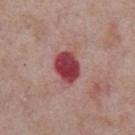Assessment: Recorded during total-body skin imaging; not selected for excision or biopsy. Image and clinical context: The lesion-visualizer software estimated border irregularity of about 1.5 on a 0–10 scale, a color-variation rating of about 4/10, and a peripheral color-asymmetry measure near 1.5. From the chest. A male patient in their mid-70s. A close-up tile cropped from a whole-body skin photograph, about 15 mm across. About 4 mm across.